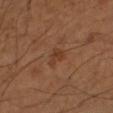The lesion was photographed on a routine skin check and not biopsied; there is no pathology result.
The lesion is located on the left forearm.
A male patient, aged approximately 45.
Cropped from a total-body skin-imaging series; the visible field is about 15 mm.